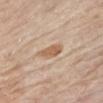notes — catalogued during a skin exam; not biopsied
subject — male, about 65 years old
image source — ~15 mm crop, total-body skin-cancer survey
anatomic site — the chest
illumination — white-light